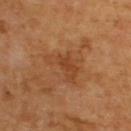Background: Cropped from a total-body skin-imaging series; the visible field is about 15 mm. The recorded lesion diameter is about 5 mm. A male patient, roughly 65 years of age. The lesion is located on the upper back.